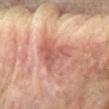Assessment:
Part of a total-body skin-imaging series; this lesion was reviewed on a skin check and was not flagged for biopsy.
Acquisition and patient details:
About 5.5 mm across. A roughly 15 mm field-of-view crop from a total-body skin photograph. An algorithmic analysis of the crop reported two-axis asymmetry of about 0.45. The software also gave a color-variation rating of about 5.5/10. A male patient, aged 68–72. The lesion is on the left forearm.